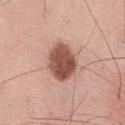The lesion was tiled from a total-body skin photograph and was not biopsied. A male patient, aged 68 to 72. A close-up tile cropped from a whole-body skin photograph, about 15 mm across. Measured at roughly 4.5 mm in maximum diameter. The tile uses white-light illumination. From the right thigh.